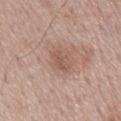Assessment:
Recorded during total-body skin imaging; not selected for excision or biopsy.
Context:
A 15 mm close-up extracted from a 3D total-body photography capture. About 3.5 mm across. Automated tile analysis of the lesion measured a mean CIELAB color near L≈54 a*≈20 b*≈25, a lesion–skin lightness drop of about 8, and a normalized lesion–skin contrast near 5.5. It also reported a classifier nevus-likeness of about 0/100 and a detector confidence of about 100 out of 100 that the crop contains a lesion. The lesion is located on the back. A male patient, aged around 70.